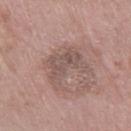The lesion was photographed on a routine skin check and not biopsied; there is no pathology result. Located on the right thigh. Automated tile analysis of the lesion measured a footprint of about 13 mm², an eccentricity of roughly 0.8, and two-axis asymmetry of about 0.45. And it measured a border-irregularity rating of about 6/10, internal color variation of about 3 on a 0–10 scale, and a peripheral color-asymmetry measure near 1. A female subject, aged approximately 75. The recorded lesion diameter is about 5 mm. A lesion tile, about 15 mm wide, cut from a 3D total-body photograph.